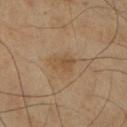Case summary:
* follow-up · imaged on a skin check; not biopsied
* patient · male, aged 68 to 72
* image source · 15 mm crop, total-body photography
* anatomic site · the right lower leg